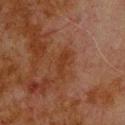Recorded during total-body skin imaging; not selected for excision or biopsy. Automated image analysis of the tile measured an average lesion color of about L≈27 a*≈20 b*≈27 (CIELAB), a lesion–skin lightness drop of about 5, and a normalized lesion–skin contrast near 6.5. Approximately 3 mm at its widest. From the upper back. A 15 mm close-up extracted from a 3D total-body photography capture. Captured under cross-polarized illumination. A male subject, roughly 80 years of age.